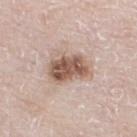Impression:
Imaged during a routine full-body skin examination; the lesion was not biopsied and no histopathology is available.
Acquisition and patient details:
A male subject, aged 78–82. The lesion is located on the left lower leg. An algorithmic analysis of the crop reported a lesion area of about 12 mm², an eccentricity of roughly 0.75, and a shape-asymmetry score of about 0.15 (0 = symmetric). It also reported a border-irregularity index near 2/10 and radial color variation of about 2. The analysis additionally found an automated nevus-likeness rating near 70 out of 100 and a detector confidence of about 100 out of 100 that the crop contains a lesion. A lesion tile, about 15 mm wide, cut from a 3D total-body photograph. This is a white-light tile. About 4.5 mm across.